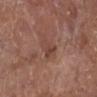Clinical impression:
Captured during whole-body skin photography for melanoma surveillance; the lesion was not biopsied.
Context:
A female patient, about 80 years old. About 3 mm across. On the left lower leg. A region of skin cropped from a whole-body photographic capture, roughly 15 mm wide. Captured under white-light illumination.